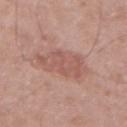Notes:
* notes — no biopsy performed (imaged during a skin exam)